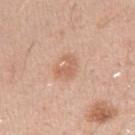notes = imaged on a skin check; not biopsied | patient = male, roughly 30 years of age | diameter = ≈3 mm | image = 15 mm crop, total-body photography | lighting = white-light | image-analysis metrics = an eccentricity of roughly 0.7 and a symmetry-axis asymmetry near 0.45; a within-lesion color-variation index near 1.5/10 and radial color variation of about 0.5; a classifier nevus-likeness of about 20/100 and lesion-presence confidence of about 100/100 | location = the arm.